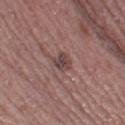Imaged during a routine full-body skin examination; the lesion was not biopsied and no histopathology is available.
The lesion is on the left thigh.
A male subject, in their mid-60s.
Cropped from a total-body skin-imaging series; the visible field is about 15 mm.